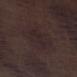Assessment:
Part of a total-body skin-imaging series; this lesion was reviewed on a skin check and was not flagged for biopsy.
Image and clinical context:
This image is a 15 mm lesion crop taken from a total-body photograph. The tile uses white-light illumination. On the leg. Approximately 6 mm at its widest. A male patient, aged around 70.